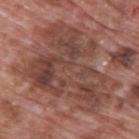This lesion was catalogued during total-body skin photography and was not selected for biopsy.
A male patient, aged 68–72.
The tile uses white-light illumination.
The lesion is located on the upper back.
A region of skin cropped from a whole-body photographic capture, roughly 15 mm wide.